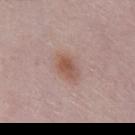A female subject, aged around 65.
The lesion is on the chest.
This image is a 15 mm lesion crop taken from a total-body photograph.